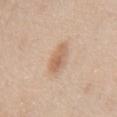workup: imaged on a skin check; not biopsied | patient: male, aged approximately 60 | image: 15 mm crop, total-body photography | body site: the mid back.